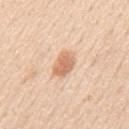Q: Was a biopsy performed?
A: total-body-photography surveillance lesion; no biopsy
Q: How was this image acquired?
A: 15 mm crop, total-body photography
Q: What is the anatomic site?
A: the mid back
Q: Lesion size?
A: about 3.5 mm
Q: Who is the patient?
A: male, aged approximately 60
Q: Illumination type?
A: white-light illumination
Q: Automated lesion metrics?
A: an eccentricity of roughly 0.8 and a symmetry-axis asymmetry near 0.15; a border-irregularity index near 2/10 and a peripheral color-asymmetry measure near 0.5